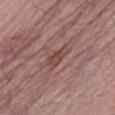Impression: Captured during whole-body skin photography for melanoma surveillance; the lesion was not biopsied. Image and clinical context: The lesion's longest dimension is about 3.5 mm. The tile uses white-light illumination. Automated image analysis of the tile measured a footprint of about 5.5 mm², an eccentricity of roughly 0.85, and two-axis asymmetry of about 0.3. The analysis additionally found a mean CIELAB color near L≈46 a*≈20 b*≈23, about 7 CIELAB-L* units darker than the surrounding skin, and a lesion-to-skin contrast of about 6 (normalized; higher = more distinct). And it measured a detector confidence of about 85 out of 100 that the crop contains a lesion. A roughly 15 mm field-of-view crop from a total-body skin photograph. A male patient aged approximately 70. On the abdomen.